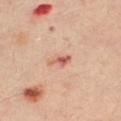Impression: The lesion was tiled from a total-body skin photograph and was not biopsied. Background: From the right leg. The lesion's longest dimension is about 2.5 mm. A female patient aged 38–42. A 15 mm close-up extracted from a 3D total-body photography capture. The tile uses cross-polarized illumination.